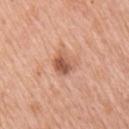Imaged during a routine full-body skin examination; the lesion was not biopsied and no histopathology is available. Imaged with white-light lighting. A close-up tile cropped from a whole-body skin photograph, about 15 mm across. Located on the right upper arm. About 3 mm across. The patient is a female in their mid-40s.